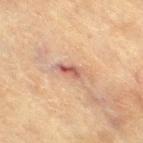  biopsy_status: not biopsied; imaged during a skin examination
  image:
    source: total-body photography crop
    field_of_view_mm: 15
  site: right thigh
  patient:
    sex: female
    age_approx: 80
  lighting: cross-polarized